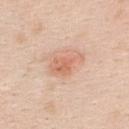Findings:
- workup · imaged on a skin check; not biopsied
- image source · 15 mm crop, total-body photography
- lighting · white-light illumination
- patient · female, about 40 years old
- automated lesion analysis · about 9 CIELAB-L* units darker than the surrounding skin and a normalized lesion–skin contrast near 6; a border-irregularity index near 4/10, a color-variation rating of about 3/10, and peripheral color asymmetry of about 1; a nevus-likeness score of about 70/100 and lesion-presence confidence of about 100/100
- anatomic site · the upper back
- lesion size · about 4 mm